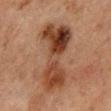Recorded during total-body skin imaging; not selected for excision or biopsy. Located on the abdomen. Cropped from a total-body skin-imaging series; the visible field is about 15 mm. A male patient in their mid- to late 60s.